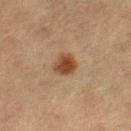biopsy_status: not biopsied; imaged during a skin examination
lighting: cross-polarized
patient:
  sex: female
  age_approx: 55
lesion_size:
  long_diameter_mm_approx: 3.0
image:
  source: total-body photography crop
  field_of_view_mm: 15
site: right lower leg